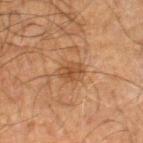– biopsy status · imaged on a skin check; not biopsied
– patient · male, in their 70s
– automated lesion analysis · a border-irregularity index near 3/10 and a within-lesion color-variation index near 2.5/10
– image · ~15 mm tile from a whole-body skin photo
– diameter · ~2.5 mm (longest diameter)
– location · the right thigh